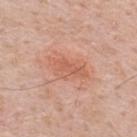The lesion was tiled from a total-body skin photograph and was not biopsied.
The lesion's longest dimension is about 5 mm.
The lesion is located on the back.
A 15 mm crop from a total-body photograph taken for skin-cancer surveillance.
The patient is a male aged approximately 50.
Imaged with white-light lighting.